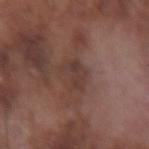This lesion was catalogued during total-body skin photography and was not selected for biopsy. A male subject aged 73 to 77. A region of skin cropped from a whole-body photographic capture, roughly 15 mm wide. This is a white-light tile. Located on the right forearm. An algorithmic analysis of the crop reported a border-irregularity index near 4.5/10, a color-variation rating of about 2.5/10, and peripheral color asymmetry of about 1. It also reported an automated nevus-likeness rating near 0 out of 100.